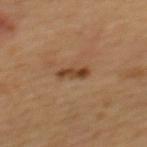{
  "biopsy_status": "not biopsied; imaged during a skin examination",
  "site": "mid back",
  "image": {
    "source": "total-body photography crop",
    "field_of_view_mm": 15
  },
  "patient": {
    "sex": "male",
    "age_approx": 60
  },
  "lesion_size": {
    "long_diameter_mm_approx": 3.5
  },
  "automated_metrics": {
    "area_mm2_approx": 4.0,
    "shape_asymmetry": 0.3,
    "border_irregularity_0_10": 3.5,
    "color_variation_0_10": 2.0,
    "peripheral_color_asymmetry": 0.0,
    "nevus_likeness_0_100": 75,
    "lesion_detection_confidence_0_100": 100
  }
}